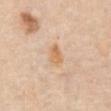| field | value |
|---|---|
| biopsy status | catalogued during a skin exam; not biopsied |
| illumination | white-light |
| body site | the abdomen |
| acquisition | ~15 mm crop, total-body skin-cancer survey |
| lesion size | about 2.5 mm |
| patient | female, in their mid- to late 60s |
| automated lesion analysis | a footprint of about 4 mm² and a symmetry-axis asymmetry near 0.2; a lesion color around L≈67 a*≈20 b*≈39 in CIELAB and roughly 8 lightness units darker than nearby skin; border irregularity of about 2 on a 0–10 scale, internal color variation of about 2.5 on a 0–10 scale, and a peripheral color-asymmetry measure near 1 |